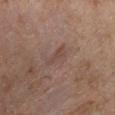Q: Was this lesion biopsied?
A: catalogued during a skin exam; not biopsied
Q: Patient demographics?
A: female, roughly 40 years of age
Q: How was the tile lit?
A: cross-polarized
Q: Where on the body is the lesion?
A: the right lower leg
Q: Automated lesion metrics?
A: an area of roughly 4 mm², an outline eccentricity of about 0.85 (0 = round, 1 = elongated), and two-axis asymmetry of about 0.45; a lesion color around L≈45 a*≈17 b*≈23 in CIELAB, about 6 CIELAB-L* units darker than the surrounding skin, and a lesion-to-skin contrast of about 5 (normalized; higher = more distinct); a nevus-likeness score of about 0/100 and a lesion-detection confidence of about 100/100
Q: How was this image acquired?
A: ~15 mm crop, total-body skin-cancer survey